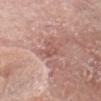Impression:
The lesion was photographed on a routine skin check and not biopsied; there is no pathology result.
Context:
A male patient aged 63–67. Captured under white-light illumination. This image is a 15 mm lesion crop taken from a total-body photograph. The total-body-photography lesion software estimated a shape eccentricity near 0.8 and a shape-asymmetry score of about 0.35 (0 = symmetric). The analysis additionally found a lesion color around L≈55 a*≈23 b*≈24 in CIELAB, roughly 8 lightness units darker than nearby skin, and a normalized border contrast of about 5.5. And it measured border irregularity of about 4 on a 0–10 scale, a color-variation rating of about 2.5/10, and a peripheral color-asymmetry measure near 1. It also reported a nevus-likeness score of about 0/100 and a detector confidence of about 95 out of 100 that the crop contains a lesion. The lesion's longest dimension is about 3.5 mm. The lesion is on the head or neck.